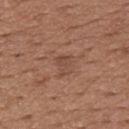The lesion was photographed on a routine skin check and not biopsied; there is no pathology result.